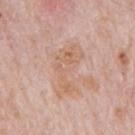follow-up: no biopsy performed (imaged during a skin exam) | lesion diameter: ≈6 mm | illumination: white-light | subject: male, in their 80s | image: ~15 mm crop, total-body skin-cancer survey | body site: the mid back.